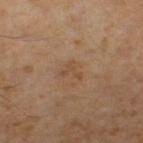Recorded during total-body skin imaging; not selected for excision or biopsy.
A male patient, in their 60s.
Captured under cross-polarized illumination.
Automated tile analysis of the lesion measured a footprint of about 2.5 mm², a shape eccentricity near 0.8, and two-axis asymmetry of about 0.65. It also reported a nevus-likeness score of about 0/100 and lesion-presence confidence of about 100/100.
The lesion is on the right lower leg.
A region of skin cropped from a whole-body photographic capture, roughly 15 mm wide.